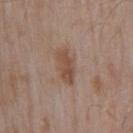Assessment:
No biopsy was performed on this lesion — it was imaged during a full skin examination and was not determined to be concerning.
Acquisition and patient details:
Cropped from a whole-body photographic skin survey; the tile spans about 15 mm. The recorded lesion diameter is about 4 mm. Automated tile analysis of the lesion measured a classifier nevus-likeness of about 0/100. Captured under white-light illumination. A male subject, aged around 55. The lesion is located on the right upper arm.